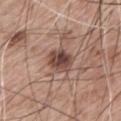Case summary:
* follow-up · no biopsy performed (imaged during a skin exam)
* subject · male, about 60 years old
* lesion size · ~3.5 mm (longest diameter)
* anatomic site · the mid back
* image source · total-body-photography crop, ~15 mm field of view